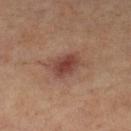{
  "biopsy_status": "not biopsied; imaged during a skin examination"
}